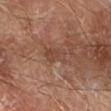follow-up: no biopsy performed (imaged during a skin exam); body site: the right forearm; patient: male, aged 68–72; image: ~15 mm crop, total-body skin-cancer survey.